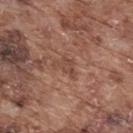Captured during whole-body skin photography for melanoma surveillance; the lesion was not biopsied.